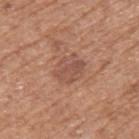{
  "biopsy_status": "not biopsied; imaged during a skin examination",
  "site": "left upper arm",
  "lighting": "white-light",
  "image": {
    "source": "total-body photography crop",
    "field_of_view_mm": 15
  },
  "patient": {
    "sex": "male",
    "age_approx": 75
  },
  "lesion_size": {
    "long_diameter_mm_approx": 3.5
  },
  "automated_metrics": {
    "eccentricity": 0.6,
    "shape_asymmetry": 0.2
  }
}